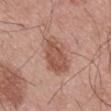patient:
  sex: male
  age_approx: 55
lighting: white-light
image:
  source: total-body photography crop
  field_of_view_mm: 15
lesion_size:
  long_diameter_mm_approx: 6.5
site: mid back